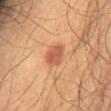No biopsy was performed on this lesion — it was imaged during a full skin examination and was not determined to be concerning.
Located on the abdomen.
The recorded lesion diameter is about 3.5 mm.
A male subject, in their 60s.
A region of skin cropped from a whole-body photographic capture, roughly 15 mm wide.
The total-body-photography lesion software estimated a mean CIELAB color near L≈52 a*≈26 b*≈33, about 10 CIELAB-L* units darker than the surrounding skin, and a normalized lesion–skin contrast near 7. The software also gave a border-irregularity index near 2/10, a color-variation rating of about 3/10, and a peripheral color-asymmetry measure near 1.